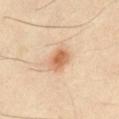Impression:
Captured during whole-body skin photography for melanoma surveillance; the lesion was not biopsied.
Background:
A 15 mm close-up tile from a total-body photography series done for melanoma screening. This is a cross-polarized tile. On the abdomen. A male subject, about 50 years old. The lesion-visualizer software estimated a shape eccentricity near 0.7. The software also gave a lesion color around L≈63 a*≈22 b*≈36 in CIELAB and about 12 CIELAB-L* units darker than the surrounding skin. And it measured border irregularity of about 1.5 on a 0–10 scale, a within-lesion color-variation index near 4/10, and peripheral color asymmetry of about 1. The recorded lesion diameter is about 3 mm.